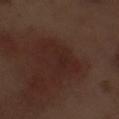biopsy_status: not biopsied; imaged during a skin examination
patient:
  sex: male
  age_approx: 70
site: left forearm
lesion_size:
  long_diameter_mm_approx: 4.5
lighting: white-light
image:
  source: total-body photography crop
  field_of_view_mm: 15